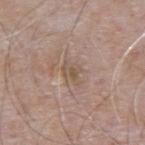Part of a total-body skin-imaging series; this lesion was reviewed on a skin check and was not flagged for biopsy. Imaged with white-light lighting. From the upper back. A 15 mm close-up extracted from a 3D total-body photography capture. The patient is a male in their mid- to late 60s.